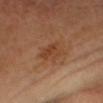Imaged with cross-polarized lighting.
Longest diameter approximately 3.5 mm.
On the head or neck.
A 15 mm close-up extracted from a 3D total-body photography capture.
Automated image analysis of the tile measured a footprint of about 7 mm², an outline eccentricity of about 0.6 (0 = round, 1 = elongated), and two-axis asymmetry of about 0.25. And it measured an average lesion color of about L≈42 a*≈22 b*≈34 (CIELAB) and about 6 CIELAB-L* units darker than the surrounding skin. The analysis additionally found a border-irregularity rating of about 2.5/10 and a peripheral color-asymmetry measure near 1. The analysis additionally found a nevus-likeness score of about 0/100 and a lesion-detection confidence of about 100/100.
A male subject, aged 38–42.